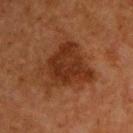  biopsy_status: not biopsied; imaged during a skin examination
  lesion_size:
    long_diameter_mm_approx: 6.5
  patient:
    sex: male
    age_approx: 65
  automated_metrics:
    area_mm2_approx: 21.0
    shape_asymmetry: 0.35
    vs_skin_darker_L: 9.0
    vs_skin_contrast_norm: 8.5
    nevus_likeness_0_100: 45
    lesion_detection_confidence_0_100: 100
  lighting: cross-polarized
  image:
    source: total-body photography crop
    field_of_view_mm: 15
  site: upper back